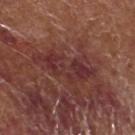Impression: This lesion was catalogued during total-body skin photography and was not selected for biopsy. Clinical summary: The subject is a male in their mid-70s. The lesion is on the left lower leg. A region of skin cropped from a whole-body photographic capture, roughly 15 mm wide. Imaged with white-light lighting. Approximately 7.5 mm at its widest.